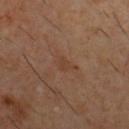Findings:
- biopsy status: imaged on a skin check; not biopsied
- image-analysis metrics: an outline eccentricity of about 0.75 (0 = round, 1 = elongated) and a shape-asymmetry score of about 0.4 (0 = symmetric); a classifier nevus-likeness of about 0/100
- diameter: ≈2.5 mm
- body site: the chest
- subject: male, roughly 60 years of age
- acquisition: total-body-photography crop, ~15 mm field of view